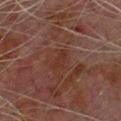Q: Is there a histopathology result?
A: no biopsy performed (imaged during a skin exam)
Q: Where on the body is the lesion?
A: the chest
Q: How was this image acquired?
A: ~15 mm tile from a whole-body skin photo
Q: What did automated image analysis measure?
A: an area of roughly 3.5 mm², a shape eccentricity near 0.85, and a symmetry-axis asymmetry near 0.4; about 4 CIELAB-L* units darker than the surrounding skin; border irregularity of about 4 on a 0–10 scale, internal color variation of about 1.5 on a 0–10 scale, and a peripheral color-asymmetry measure near 1
Q: What lighting was used for the tile?
A: cross-polarized illumination
Q: How large is the lesion?
A: ~3 mm (longest diameter)
Q: Who is the patient?
A: male, aged 78 to 82